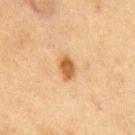This lesion was catalogued during total-body skin photography and was not selected for biopsy.
A close-up tile cropped from a whole-body skin photograph, about 15 mm across.
A male patient, about 75 years old.
The lesion is on the chest.